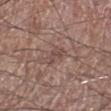Clinical summary: Imaged with white-light lighting. The lesion is on the right lower leg. Automated image analysis of the tile measured a footprint of about 4 mm², an outline eccentricity of about 0.8 (0 = round, 1 = elongated), and a symmetry-axis asymmetry near 0.45. The software also gave a lesion color around L≈47 a*≈17 b*≈22 in CIELAB and a lesion–skin lightness drop of about 7. And it measured internal color variation of about 1.5 on a 0–10 scale and peripheral color asymmetry of about 0.5. The subject is a male in their mid-70s. A lesion tile, about 15 mm wide, cut from a 3D total-body photograph.